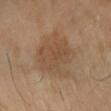Imaged during a routine full-body skin examination; the lesion was not biopsied and no histopathology is available. A 15 mm crop from a total-body photograph taken for skin-cancer surveillance. The lesion-visualizer software estimated an area of roughly 23 mm², an eccentricity of roughly 0.6, and a symmetry-axis asymmetry near 0.25. It also reported an automated nevus-likeness rating near 0 out of 100 and a detector confidence of about 100 out of 100 that the crop contains a lesion. A female patient, in their 70s. The lesion is on the right lower leg. The recorded lesion diameter is about 6.5 mm. Captured under cross-polarized illumination.